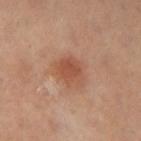workup — catalogued during a skin exam; not biopsied
site — the left thigh
automated metrics — a lesion area of about 7.5 mm², a shape eccentricity near 0.6, and two-axis asymmetry of about 0.25; a border-irregularity rating of about 2/10 and radial color variation of about 1
image — 15 mm crop, total-body photography
diameter — ~3.5 mm (longest diameter)
patient — female, in their 50s